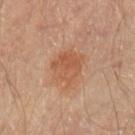  biopsy_status: not biopsied; imaged during a skin examination
  image:
    source: total-body photography crop
    field_of_view_mm: 15
  patient:
    sex: male
    age_approx: 65
  automated_metrics:
    cielab_L: 53
    cielab_a: 22
    cielab_b: 33
    vs_skin_darker_L: 8.0
    vs_skin_contrast_norm: 6.0
    border_irregularity_0_10: 3.0
    color_variation_0_10: 3.5
    peripheral_color_asymmetry: 1.0
  lesion_size:
    long_diameter_mm_approx: 4.0
  lighting: cross-polarized
  site: arm